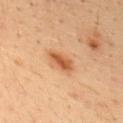follow-up — total-body-photography surveillance lesion; no biopsy | illumination — cross-polarized illumination | lesion size — ≈3.5 mm | subject — male, roughly 35 years of age | body site — the upper back | imaging modality — ~15 mm crop, total-body skin-cancer survey.